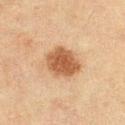Impression: Part of a total-body skin-imaging series; this lesion was reviewed on a skin check and was not flagged for biopsy. Context: A lesion tile, about 15 mm wide, cut from a 3D total-body photograph. Imaged with cross-polarized lighting. The lesion is located on the left thigh. A female subject aged around 55. Approximately 4 mm at its widest.